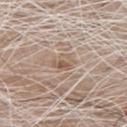Captured during whole-body skin photography for melanoma surveillance; the lesion was not biopsied.